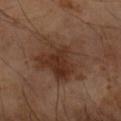follow-up: no biopsy performed (imaged during a skin exam); acquisition: total-body-photography crop, ~15 mm field of view; lesion size: ≈7 mm; anatomic site: the right forearm; lighting: cross-polarized; subject: male, aged 63–67.